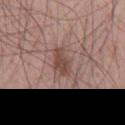| key | value |
|---|---|
| patient | male, aged 53 to 57 |
| lesion size | ≈3.5 mm |
| acquisition | total-body-photography crop, ~15 mm field of view |
| anatomic site | the chest |
| TBP lesion metrics | a border-irregularity rating of about 2.5/10, a color-variation rating of about 2.5/10, and radial color variation of about 0.5; an automated nevus-likeness rating near 60 out of 100 and a detector confidence of about 100 out of 100 that the crop contains a lesion |